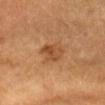Assessment:
No biopsy was performed on this lesion — it was imaged during a full skin examination and was not determined to be concerning.
Context:
A 15 mm close-up extracted from a 3D total-body photography capture. Located on the head or neck. The lesion's longest dimension is about 3 mm. This is a cross-polarized tile. An algorithmic analysis of the crop reported a lesion color around L≈48 a*≈23 b*≈37 in CIELAB, roughly 9 lightness units darker than nearby skin, and a lesion-to-skin contrast of about 6.5 (normalized; higher = more distinct). And it measured a classifier nevus-likeness of about 15/100. A female patient in their mid- to late 60s.